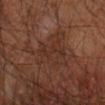* lesion diameter: ~5.5 mm (longest diameter)
* image: ~15 mm tile from a whole-body skin photo
* site: the left arm
* subject: male, aged 63–67